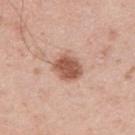{"biopsy_status": "not biopsied; imaged during a skin examination", "site": "right upper arm", "patient": {"sex": "male", "age_approx": 40}, "image": {"source": "total-body photography crop", "field_of_view_mm": 15}, "automated_metrics": {"area_mm2_approx": 8.5, "eccentricity": 0.45, "shape_asymmetry": 0.15, "nevus_likeness_0_100": 95, "lesion_detection_confidence_0_100": 100}}